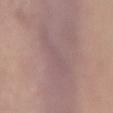workup: catalogued during a skin exam; not biopsied
location: the abdomen
image: total-body-photography crop, ~15 mm field of view
lesion diameter: ~4.5 mm (longest diameter)
image-analysis metrics: a lesion area of about 8 mm², a shape eccentricity near 0.85, and a symmetry-axis asymmetry near 0.45
patient: female, aged 63–67